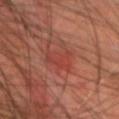biopsy status = imaged on a skin check; not biopsied
subject = male, in their 70s
image source = total-body-photography crop, ~15 mm field of view
location = the head or neck
TBP lesion metrics = an eccentricity of roughly 0.85 and a symmetry-axis asymmetry near 0.3